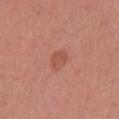biopsy_status: not biopsied; imaged during a skin examination
site: mid back
lighting: white-light
patient:
  sex: male
  age_approx: 45
image:
  source: total-body photography crop
  field_of_view_mm: 15